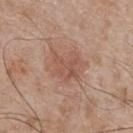Part of a total-body skin-imaging series; this lesion was reviewed on a skin check and was not flagged for biopsy. The lesion is on the chest. Longest diameter approximately 4 mm. A 15 mm close-up tile from a total-body photography series done for melanoma screening. This is a white-light tile. A male patient, roughly 65 years of age. The total-body-photography lesion software estimated an average lesion color of about L≈53 a*≈21 b*≈28 (CIELAB), about 7 CIELAB-L* units darker than the surrounding skin, and a lesion-to-skin contrast of about 5.5 (normalized; higher = more distinct). The analysis additionally found a color-variation rating of about 2.5/10 and a peripheral color-asymmetry measure near 1.